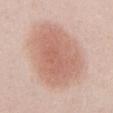Impression: No biopsy was performed on this lesion — it was imaged during a full skin examination and was not determined to be concerning.